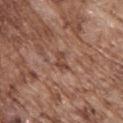illumination = white-light illumination
image-analysis metrics = a lesion area of about 3.5 mm², an eccentricity of roughly 0.85, and two-axis asymmetry of about 0.35; a mean CIELAB color near L≈45 a*≈21 b*≈27 and a normalized border contrast of about 6.5; a border-irregularity index near 4/10 and a within-lesion color-variation index near 1/10
site = the upper back
image source = ~15 mm tile from a whole-body skin photo
patient = male, roughly 75 years of age
lesion size = ≈2.5 mm